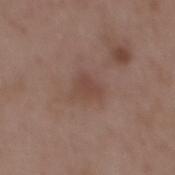follow-up: no biopsy performed (imaged during a skin exam) | illumination: white-light illumination | patient: male, aged 48 to 52 | location: the mid back | automated lesion analysis: a footprint of about 4 mm², an outline eccentricity of about 0.65 (0 = round, 1 = elongated), and a shape-asymmetry score of about 0.3 (0 = symmetric); a border-irregularity index near 2.5/10 and a peripheral color-asymmetry measure near 0.5; an automated nevus-likeness rating near 0 out of 100 and lesion-presence confidence of about 100/100 | acquisition: ~15 mm tile from a whole-body skin photo.